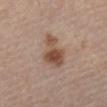Impression: The lesion was photographed on a routine skin check and not biopsied; there is no pathology result. Background: A male subject, aged 48–52. The lesion is located on the left leg. Cropped from a total-body skin-imaging series; the visible field is about 15 mm.